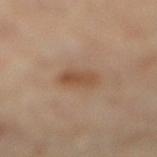Notes:
* workup: total-body-photography surveillance lesion; no biopsy
* tile lighting: cross-polarized illumination
* imaging modality: total-body-photography crop, ~15 mm field of view
* size: ~3 mm (longest diameter)
* anatomic site: the right lower leg
* automated metrics: an area of roughly 6 mm² and a shape-asymmetry score of about 0.15 (0 = symmetric); a lesion color around L≈49 a*≈18 b*≈31 in CIELAB; a classifier nevus-likeness of about 75/100
* subject: female, about 60 years old